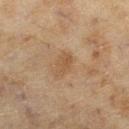Clinical impression: No biopsy was performed on this lesion — it was imaged during a full skin examination and was not determined to be concerning. Context: Automated tile analysis of the lesion measured a lesion area of about 4 mm², an eccentricity of roughly 0.75, and a shape-asymmetry score of about 0.2 (0 = symmetric). The software also gave a lesion color around L≈48 a*≈16 b*≈32 in CIELAB and a normalized border contrast of about 6. The software also gave an automated nevus-likeness rating near 0 out of 100 and a lesion-detection confidence of about 100/100. On the leg. The subject is a female aged 58 to 62. Cropped from a total-body skin-imaging series; the visible field is about 15 mm. The recorded lesion diameter is about 2.5 mm. Imaged with cross-polarized lighting.